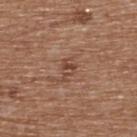The lesion was tiled from a total-body skin photograph and was not biopsied.